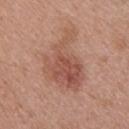Q: Was this lesion biopsied?
A: no biopsy performed (imaged during a skin exam)
Q: How large is the lesion?
A: ~8 mm (longest diameter)
Q: Illumination type?
A: white-light
Q: Who is the patient?
A: female, aged approximately 40
Q: Lesion location?
A: the upper back
Q: How was this image acquired?
A: ~15 mm tile from a whole-body skin photo